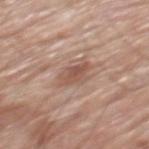• workup — imaged on a skin check; not biopsied
• illumination — white-light
• subject — male, aged approximately 80
• size — about 3 mm
• site — the mid back
• imaging modality — 15 mm crop, total-body photography